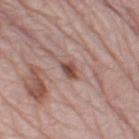No biopsy was performed on this lesion — it was imaged during a full skin examination and was not determined to be concerning. A male subject in their 80s. The tile uses white-light illumination. The lesion's longest dimension is about 2.5 mm. Cropped from a total-body skin-imaging series; the visible field is about 15 mm. An algorithmic analysis of the crop reported an area of roughly 3 mm², a shape eccentricity near 0.75, and a shape-asymmetry score of about 0.2 (0 = symmetric). It also reported an average lesion color of about L≈48 a*≈21 b*≈25 (CIELAB), roughly 13 lightness units darker than nearby skin, and a normalized border contrast of about 9.5. And it measured a border-irregularity rating of about 2/10, a within-lesion color-variation index near 3.5/10, and peripheral color asymmetry of about 1.5. The analysis additionally found an automated nevus-likeness rating near 75 out of 100. The lesion is located on the left thigh.